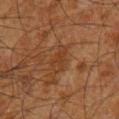The lesion was photographed on a routine skin check and not biopsied; there is no pathology result.
A male patient aged around 65.
The lesion is on the left upper arm.
A region of skin cropped from a whole-body photographic capture, roughly 15 mm wide.